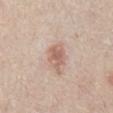Assessment:
This lesion was catalogued during total-body skin photography and was not selected for biopsy.
Background:
A close-up tile cropped from a whole-body skin photograph, about 15 mm across. Longest diameter approximately 3 mm. A male patient roughly 70 years of age. Captured under white-light illumination. The lesion is located on the abdomen. Automated image analysis of the tile measured border irregularity of about 2 on a 0–10 scale, internal color variation of about 2.5 on a 0–10 scale, and a peripheral color-asymmetry measure near 1. It also reported a nevus-likeness score of about 70/100 and a detector confidence of about 100 out of 100 that the crop contains a lesion.